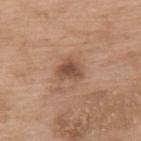Part of a total-body skin-imaging series; this lesion was reviewed on a skin check and was not flagged for biopsy.
A female patient, about 75 years old.
This is a white-light tile.
The total-body-photography lesion software estimated an area of roughly 5 mm², an outline eccentricity of about 0.65 (0 = round, 1 = elongated), and a shape-asymmetry score of about 0.3 (0 = symmetric). The analysis additionally found roughly 11 lightness units darker than nearby skin and a normalized border contrast of about 8. The software also gave an automated nevus-likeness rating near 40 out of 100 and a detector confidence of about 100 out of 100 that the crop contains a lesion.
The lesion is on the upper back.
Cropped from a total-body skin-imaging series; the visible field is about 15 mm.